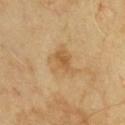| key | value |
|---|---|
| biopsy status | total-body-photography surveillance lesion; no biopsy |
| lighting | cross-polarized |
| image | 15 mm crop, total-body photography |
| diameter | ~4.5 mm (longest diameter) |
| location | the chest |
| subject | male, roughly 65 years of age |